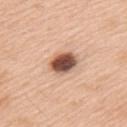follow-up=catalogued during a skin exam; not biopsied.